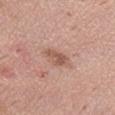Assessment:
This lesion was catalogued during total-body skin photography and was not selected for biopsy.
Context:
The patient is a female about 40 years old. A 15 mm close-up extracted from a 3D total-body photography capture. The lesion is located on the abdomen. About 3 mm across. Imaged with white-light lighting.